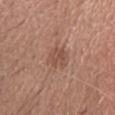Assessment:
Part of a total-body skin-imaging series; this lesion was reviewed on a skin check and was not flagged for biopsy.
Clinical summary:
Captured under white-light illumination. The subject is a female roughly 60 years of age. This image is a 15 mm lesion crop taken from a total-body photograph. The lesion's longest dimension is about 3 mm. On the head or neck. Automated tile analysis of the lesion measured an average lesion color of about L≈50 a*≈21 b*≈27 (CIELAB) and roughly 8 lightness units darker than nearby skin.